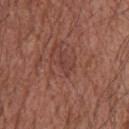The lesion was photographed on a routine skin check and not biopsied; there is no pathology result. A male subject aged approximately 65. Located on the upper back. The tile uses white-light illumination. Longest diameter approximately 3 mm. A close-up tile cropped from a whole-body skin photograph, about 15 mm across.